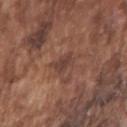follow-up: no biopsy performed (imaged during a skin exam) | anatomic site: the right upper arm | lesion diameter: ~3 mm (longest diameter) | subject: male, about 75 years old | acquisition: ~15 mm crop, total-body skin-cancer survey | image-analysis metrics: border irregularity of about 4 on a 0–10 scale and a within-lesion color-variation index near 2/10 | lighting: white-light.